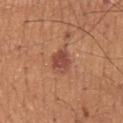Case summary:
* notes: no biopsy performed (imaged during a skin exam)
* automated metrics: a footprint of about 6 mm² and a shape eccentricity near 0.6; a lesion color around L≈48 a*≈26 b*≈29 in CIELAB, a lesion–skin lightness drop of about 10, and a normalized border contrast of about 8
* subject: male, aged 53–57
* lighting: white-light
* body site: the mid back
* diameter: ≈3 mm
* imaging modality: ~15 mm tile from a whole-body skin photo